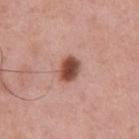Recorded during total-body skin imaging; not selected for excision or biopsy. The lesion is located on the chest. This is a white-light tile. A 15 mm close-up extracted from a 3D total-body photography capture. Measured at roughly 3 mm in maximum diameter. The lesion-visualizer software estimated a lesion area of about 6 mm², a shape eccentricity near 0.65, and a shape-asymmetry score of about 0.2 (0 = symmetric). And it measured about 17 CIELAB-L* units darker than the surrounding skin and a lesion-to-skin contrast of about 11.5 (normalized; higher = more distinct). And it measured a border-irregularity rating of about 1.5/10, internal color variation of about 6 on a 0–10 scale, and a peripheral color-asymmetry measure near 2. A male patient aged around 60.